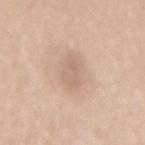Notes:
* notes · imaged on a skin check; not biopsied
* subject · female, aged approximately 70
* image source · 15 mm crop, total-body photography
* anatomic site · the lower back